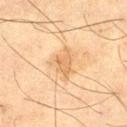Notes:
* biopsy status: total-body-photography surveillance lesion; no biopsy
* location: the right thigh
* lighting: cross-polarized illumination
* image: ~15 mm tile from a whole-body skin photo
* patient: male, aged around 65